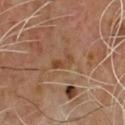Assessment: Imaged during a routine full-body skin examination; the lesion was not biopsied and no histopathology is available. Background: Captured under cross-polarized illumination. Automated image analysis of the tile measured an area of roughly 3 mm², an outline eccentricity of about 0.9 (0 = round, 1 = elongated), and a shape-asymmetry score of about 0.35 (0 = symmetric). And it measured a lesion color around L≈42 a*≈19 b*≈32 in CIELAB, about 7 CIELAB-L* units darker than the surrounding skin, and a normalized lesion–skin contrast near 6.5. The analysis additionally found a classifier nevus-likeness of about 0/100 and lesion-presence confidence of about 100/100. Located on the chest. The recorded lesion diameter is about 3 mm. A 15 mm close-up tile from a total-body photography series done for melanoma screening. The subject is a male aged 63 to 67.